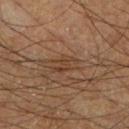follow-up — total-body-photography surveillance lesion; no biopsy | tile lighting — cross-polarized | lesion diameter — ≈3.5 mm | subject — male, aged 58–62 | body site — the right lower leg | image — ~15 mm tile from a whole-body skin photo.